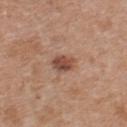Captured during whole-body skin photography for melanoma surveillance; the lesion was not biopsied. A female subject, approximately 40 years of age. The lesion is located on the chest. A region of skin cropped from a whole-body photographic capture, roughly 15 mm wide.